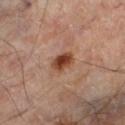Assessment:
Recorded during total-body skin imaging; not selected for excision or biopsy.
Acquisition and patient details:
This is a cross-polarized tile. Measured at roughly 2.5 mm in maximum diameter. The subject is a male in their mid-60s. The total-body-photography lesion software estimated border irregularity of about 2 on a 0–10 scale, a within-lesion color-variation index near 4/10, and radial color variation of about 1.5. The software also gave an automated nevus-likeness rating near 95 out of 100 and a lesion-detection confidence of about 100/100. A 15 mm crop from a total-body photograph taken for skin-cancer surveillance. The lesion is located on the left lower leg.